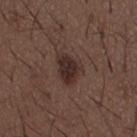<tbp_lesion>
<biopsy_status>not biopsied; imaged during a skin examination</biopsy_status>
<automated_metrics>
  <area_mm2_approx>7.5</area_mm2_approx>
  <eccentricity>0.65</eccentricity>
  <shape_asymmetry>0.3</shape_asymmetry>
  <color_variation_0_10>2.5</color_variation_0_10>
  <peripheral_color_asymmetry>0.5</peripheral_color_asymmetry>
</automated_metrics>
<site>mid back</site>
<patient>
  <sex>male</sex>
  <age_approx>50</age_approx>
</patient>
<image>
  <source>total-body photography crop</source>
  <field_of_view_mm>15</field_of_view_mm>
</image>
<lighting>white-light</lighting>
</tbp_lesion>